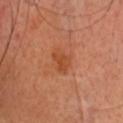Part of a total-body skin-imaging series; this lesion was reviewed on a skin check and was not flagged for biopsy. The lesion-visualizer software estimated an area of roughly 4.5 mm², an eccentricity of roughly 0.65, and a symmetry-axis asymmetry near 0.3. The software also gave border irregularity of about 2.5 on a 0–10 scale, internal color variation of about 2.5 on a 0–10 scale, and a peripheral color-asymmetry measure near 1. The analysis additionally found a classifier nevus-likeness of about 20/100 and lesion-presence confidence of about 100/100. A roughly 15 mm field-of-view crop from a total-body skin photograph. On the head or neck. Captured under cross-polarized illumination. A male subject, roughly 70 years of age. Approximately 3 mm at its widest.